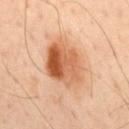Q: Is there a histopathology result?
A: imaged on a skin check; not biopsied
Q: Who is the patient?
A: male, approximately 45 years of age
Q: What is the imaging modality?
A: 15 mm crop, total-body photography
Q: What is the anatomic site?
A: the mid back